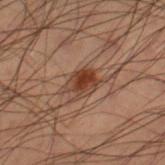Recorded during total-body skin imaging; not selected for excision or biopsy.
From the right lower leg.
This is a cross-polarized tile.
The lesion-visualizer software estimated a symmetry-axis asymmetry near 0.25. The software also gave a mean CIELAB color near L≈32 a*≈18 b*≈25 and a lesion–skin lightness drop of about 9.
A male subject aged around 55.
A 15 mm close-up extracted from a 3D total-body photography capture.